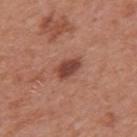| field | value |
|---|---|
| biopsy status | no biopsy performed (imaged during a skin exam) |
| body site | the mid back |
| patient | male, about 70 years old |
| image-analysis metrics | a shape eccentricity near 0.8 and two-axis asymmetry of about 0.25 |
| acquisition | ~15 mm crop, total-body skin-cancer survey |
| lesion size | ~3.5 mm (longest diameter) |
| illumination | white-light |